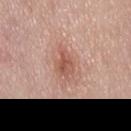The lesion was photographed on a routine skin check and not biopsied; there is no pathology result. On the mid back. Cropped from a total-body skin-imaging series; the visible field is about 15 mm. This is a white-light tile. The total-body-photography lesion software estimated an average lesion color of about L≈56 a*≈23 b*≈28 (CIELAB) and a normalized lesion–skin contrast near 7. Approximately 4 mm at its widest. A male subject, aged around 55.